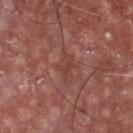This lesion was catalogued during total-body skin photography and was not selected for biopsy. Approximately 3 mm at its widest. Imaged with white-light lighting. On the chest. A male subject aged 63 to 67. A 15 mm close-up extracted from a 3D total-body photography capture.